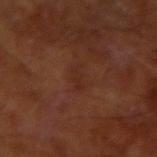Assessment: Part of a total-body skin-imaging series; this lesion was reviewed on a skin check and was not flagged for biopsy. Clinical summary: A 15 mm crop from a total-body photograph taken for skin-cancer surveillance. A male patient aged approximately 65. The lesion's longest dimension is about 3 mm. The lesion-visualizer software estimated a shape eccentricity near 0.9 and two-axis asymmetry of about 0.4. The lesion is located on the right upper arm. The tile uses cross-polarized illumination.